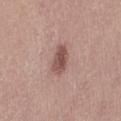* follow-up — imaged on a skin check; not biopsied
* image — total-body-photography crop, ~15 mm field of view
* site — the right thigh
* lesion size — about 3.5 mm
* subject — female, approximately 45 years of age